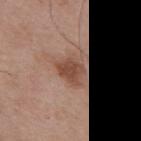<case>
  <biopsy_status>not biopsied; imaged during a skin examination</biopsy_status>
  <image>
    <source>total-body photography crop</source>
    <field_of_view_mm>15</field_of_view_mm>
  </image>
  <site>back</site>
  <lesion_size>
    <long_diameter_mm_approx>4.0</long_diameter_mm_approx>
  </lesion_size>
  <patient>
    <sex>male</sex>
    <age_approx>55</age_approx>
  </patient>
</case>